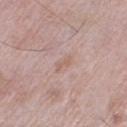{"biopsy_status": "not biopsied; imaged during a skin examination", "site": "right thigh", "automated_metrics": {"border_irregularity_0_10": 4.5}, "lesion_size": {"long_diameter_mm_approx": 2.5}, "patient": {"sex": "male", "age_approx": 75}, "image": {"source": "total-body photography crop", "field_of_view_mm": 15}}